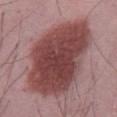Part of a total-body skin-imaging series; this lesion was reviewed on a skin check and was not flagged for biopsy.
The subject is a male roughly 50 years of age.
A 15 mm crop from a total-body photograph taken for skin-cancer surveillance.
Located on the abdomen.